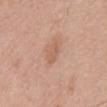{"biopsy_status": "not biopsied; imaged during a skin examination", "site": "mid back", "automated_metrics": {"area_mm2_approx": 3.5, "shape_asymmetry": 0.3, "vs_skin_darker_L": 7.0, "vs_skin_contrast_norm": 5.0, "border_irregularity_0_10": 2.5, "color_variation_0_10": 1.5, "peripheral_color_asymmetry": 0.5}, "lesion_size": {"long_diameter_mm_approx": 3.0}, "patient": {"sex": "male", "age_approx": 70}, "image": {"source": "total-body photography crop", "field_of_view_mm": 15}}